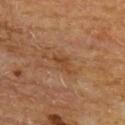Context: A lesion tile, about 15 mm wide, cut from a 3D total-body photograph. This is a cross-polarized tile. The total-body-photography lesion software estimated a footprint of about 3 mm², a shape eccentricity near 0.75, and two-axis asymmetry of about 0.45. And it measured a mean CIELAB color near L≈39 a*≈19 b*≈32, a lesion–skin lightness drop of about 6, and a lesion-to-skin contrast of about 6 (normalized; higher = more distinct). The software also gave a border-irregularity index near 4.5/10, a color-variation rating of about 1/10, and radial color variation of about 0.5. It also reported lesion-presence confidence of about 100/100. From the upper back. A male subject about 65 years old.